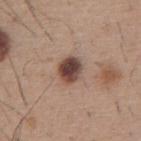| field | value |
|---|---|
| follow-up | catalogued during a skin exam; not biopsied |
| TBP lesion metrics | a footprint of about 7.5 mm² and a shape-asymmetry score of about 0.15 (0 = symmetric); a border-irregularity index near 1.5/10, internal color variation of about 6.5 on a 0–10 scale, and a peripheral color-asymmetry measure near 2 |
| lesion diameter | ~3.5 mm (longest diameter) |
| subject | male, aged approximately 60 |
| illumination | white-light illumination |
| anatomic site | the abdomen |
| imaging modality | ~15 mm tile from a whole-body skin photo |